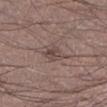<case>
  <biopsy_status>not biopsied; imaged during a skin examination</biopsy_status>
  <patient>
    <sex>male</sex>
    <age_approx>35</age_approx>
  </patient>
  <image>
    <source>total-body photography crop</source>
    <field_of_view_mm>15</field_of_view_mm>
  </image>
  <site>leg</site>
</case>